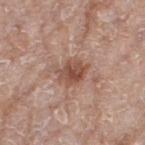Recorded during total-body skin imaging; not selected for excision or biopsy. A female patient, about 75 years old. A 15 mm close-up extracted from a 3D total-body photography capture. About 3.5 mm across. From the right thigh. The lesion-visualizer software estimated an area of roughly 7.5 mm² and a shape-asymmetry score of about 0.25 (0 = symmetric). The software also gave about 11 CIELAB-L* units darker than the surrounding skin and a lesion-to-skin contrast of about 8 (normalized; higher = more distinct).